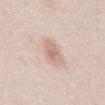| key | value |
|---|---|
| workup | no biopsy performed (imaged during a skin exam) |
| site | the abdomen |
| automated lesion analysis | a shape eccentricity near 0.7 and a shape-asymmetry score of about 0.3 (0 = symmetric); a mean CIELAB color near L≈67 a*≈19 b*≈25; a border-irregularity rating of about 2.5/10, a color-variation rating of about 3/10, and a peripheral color-asymmetry measure near 1 |
| acquisition | ~15 mm tile from a whole-body skin photo |
| tile lighting | white-light illumination |
| subject | female, aged approximately 40 |
| lesion size | ≈3 mm |